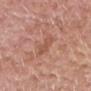The lesion was photographed on a routine skin check and not biopsied; there is no pathology result. A 15 mm crop from a total-body photograph taken for skin-cancer surveillance. An algorithmic analysis of the crop reported an area of roughly 4 mm². And it measured a border-irregularity rating of about 4/10 and a color-variation rating of about 1/10. The software also gave a classifier nevus-likeness of about 0/100. The subject is a male about 80 years old. This is a white-light tile. The lesion is located on the chest.